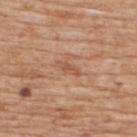follow-up: catalogued during a skin exam; not biopsied
lesion size: ~3 mm (longest diameter)
image: 15 mm crop, total-body photography
anatomic site: the upper back
tile lighting: white-light
patient: male, aged around 60